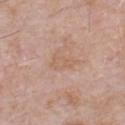<record>
  <biopsy_status>not biopsied; imaged during a skin examination</biopsy_status>
  <patient>
    <sex>male</sex>
    <age_approx>60</age_approx>
  </patient>
  <site>chest</site>
  <lighting>white-light</lighting>
  <image>
    <source>total-body photography crop</source>
    <field_of_view_mm>15</field_of_view_mm>
  </image>
  <automated_metrics>
    <area_mm2_approx>5.0</area_mm2_approx>
    <eccentricity>0.85</eccentricity>
    <cielab_L>60</cielab_L>
    <cielab_a>19</cielab_a>
    <cielab_b>30</cielab_b>
    <vs_skin_contrast_norm>4.5</vs_skin_contrast_norm>
    <border_irregularity_0_10>4.5</border_irregularity_0_10>
    <color_variation_0_10>1.5</color_variation_0_10>
    <peripheral_color_asymmetry>0.5</peripheral_color_asymmetry>
    <nevus_likeness_0_100>0</nevus_likeness_0_100>
    <lesion_detection_confidence_0_100>100</lesion_detection_confidence_0_100>
  </automated_metrics>
  <lesion_size>
    <long_diameter_mm_approx>3.5</long_diameter_mm_approx>
  </lesion_size>
</record>